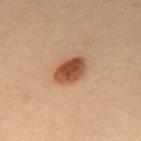The lesion was photographed on a routine skin check and not biopsied; there is no pathology result.